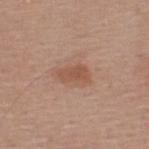biopsy status: imaged on a skin check; not biopsied
patient: male, aged 38–42
size: ~4 mm (longest diameter)
illumination: white-light
acquisition: ~15 mm tile from a whole-body skin photo
location: the upper back
image-analysis metrics: border irregularity of about 2.5 on a 0–10 scale, internal color variation of about 1.5 on a 0–10 scale, and a peripheral color-asymmetry measure near 0.5; a detector confidence of about 100 out of 100 that the crop contains a lesion